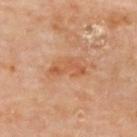| feature | finding |
|---|---|
| follow-up | catalogued during a skin exam; not biopsied |
| patient | female, approximately 60 years of age |
| imaging modality | ~15 mm crop, total-body skin-cancer survey |
| lighting | cross-polarized |
| body site | the upper back |
| image-analysis metrics | border irregularity of about 8 on a 0–10 scale, a within-lesion color-variation index near 0/10, and a peripheral color-asymmetry measure near 0; a lesion-detection confidence of about 100/100 |